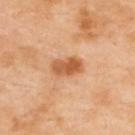* biopsy status: no biopsy performed (imaged during a skin exam)
* site: the upper back
* subject: female, in their mid- to late 40s
* image: ~15 mm crop, total-body skin-cancer survey
* illumination: cross-polarized